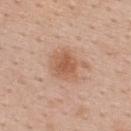Notes:
* follow-up — imaged on a skin check; not biopsied
* lesion size — ≈4 mm
* patient — female, about 40 years old
* body site — the upper back
* acquisition — 15 mm crop, total-body photography
* TBP lesion metrics — a lesion area of about 11 mm², a shape eccentricity near 0.5, and two-axis asymmetry of about 0.3
* illumination — white-light illumination